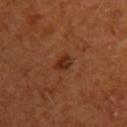subject: female, aged approximately 65
site: the left upper arm
TBP lesion metrics: a footprint of about 3.5 mm², a shape eccentricity near 0.7, and a shape-asymmetry score of about 0.2 (0 = symmetric); a mean CIELAB color near L≈29 a*≈24 b*≈31 and a lesion–skin lightness drop of about 8; internal color variation of about 2 on a 0–10 scale and peripheral color asymmetry of about 1; a nevus-likeness score of about 90/100 and lesion-presence confidence of about 100/100
acquisition: 15 mm crop, total-body photography
size: ~2.5 mm (longest diameter)
tile lighting: cross-polarized illumination